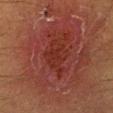Assessment:
This lesion was catalogued during total-body skin photography and was not selected for biopsy.
Clinical summary:
Approximately 4.5 mm at its widest. The patient is a male aged around 40. From the left lower leg. Automated tile analysis of the lesion measured an area of roughly 10 mm², an outline eccentricity of about 0.7 (0 = round, 1 = elongated), and a shape-asymmetry score of about 0.25 (0 = symmetric). The analysis additionally found border irregularity of about 4 on a 0–10 scale, a within-lesion color-variation index near 2/10, and a peripheral color-asymmetry measure near 0.5. Cropped from a whole-body photographic skin survey; the tile spans about 15 mm. Captured under cross-polarized illumination.